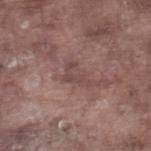{
  "biopsy_status": "not biopsied; imaged during a skin examination",
  "lesion_size": {
    "long_diameter_mm_approx": 3.0
  },
  "patient": {
    "sex": "male",
    "age_approx": 75
  },
  "site": "right lower leg",
  "automated_metrics": {
    "area_mm2_approx": 3.5,
    "eccentricity": 0.8,
    "shape_asymmetry": 0.8,
    "nevus_likeness_0_100": 0
  },
  "lighting": "white-light",
  "image": {
    "source": "total-body photography crop",
    "field_of_view_mm": 15
  }
}